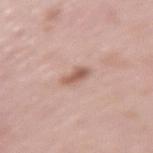Clinical impression:
This lesion was catalogued during total-body skin photography and was not selected for biopsy.
Clinical summary:
The subject is a female about 50 years old. Cropped from a total-body skin-imaging series; the visible field is about 15 mm. Longest diameter approximately 2.5 mm. From the left upper arm. Captured under white-light illumination. The lesion-visualizer software estimated about 11 CIELAB-L* units darker than the surrounding skin and a normalized lesion–skin contrast near 7.5. It also reported lesion-presence confidence of about 100/100.